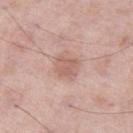Image and clinical context: The total-body-photography lesion software estimated a lesion area of about 5.5 mm² and a shape-asymmetry score of about 0.3 (0 = symmetric). It also reported an average lesion color of about L≈60 a*≈21 b*≈25 (CIELAB) and a normalized border contrast of about 5.5. It also reported a border-irregularity index near 2.5/10, internal color variation of about 2.5 on a 0–10 scale, and radial color variation of about 1. The recorded lesion diameter is about 3 mm. This image is a 15 mm lesion crop taken from a total-body photograph. The lesion is on the leg. The patient is a male aged approximately 55. This is a white-light tile.